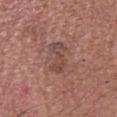Recorded during total-body skin imaging; not selected for excision or biopsy.
A lesion tile, about 15 mm wide, cut from a 3D total-body photograph.
On the head or neck.
A male subject, in their mid- to late 60s.
Automated image analysis of the tile measured a lesion area of about 8.5 mm² and a symmetry-axis asymmetry near 0.3. The analysis additionally found a lesion–skin lightness drop of about 7 and a normalized lesion–skin contrast near 5.5. The analysis additionally found a border-irregularity index near 4/10, a within-lesion color-variation index near 4.5/10, and a peripheral color-asymmetry measure near 1.5.